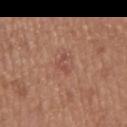Part of a total-body skin-imaging series; this lesion was reviewed on a skin check and was not flagged for biopsy.
Cropped from a total-body skin-imaging series; the visible field is about 15 mm.
The subject is a male about 65 years old.
The lesion is located on the mid back.